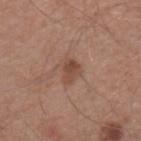<lesion>
<biopsy_status>not biopsied; imaged during a skin examination</biopsy_status>
<site>mid back</site>
<image>
  <source>total-body photography crop</source>
  <field_of_view_mm>15</field_of_view_mm>
</image>
<patient>
  <sex>male</sex>
  <age_approx>65</age_approx>
</patient>
</lesion>